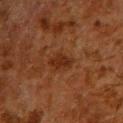Case summary:
- notes: catalogued during a skin exam; not biopsied
- lesion size: about 3 mm
- illumination: cross-polarized
- acquisition: total-body-photography crop, ~15 mm field of view
- subject: male, aged around 60
- body site: the chest
- automated lesion analysis: a border-irregularity rating of about 3/10, a within-lesion color-variation index near 2/10, and radial color variation of about 0.5; a classifier nevus-likeness of about 35/100